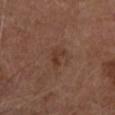follow-up: imaged on a skin check; not biopsied | imaging modality: ~15 mm tile from a whole-body skin photo | subject: male, in their mid- to late 70s | TBP lesion metrics: an eccentricity of roughly 0.75; a border-irregularity index near 5.5/10 and peripheral color asymmetry of about 0; a detector confidence of about 100 out of 100 that the crop contains a lesion | diameter: ≈2.5 mm | site: the head or neck.